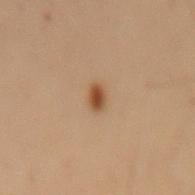Assessment:
The lesion was tiled from a total-body skin photograph and was not biopsied.
Image and clinical context:
A female patient roughly 50 years of age. The total-body-photography lesion software estimated a lesion area of about 3 mm², an eccentricity of roughly 0.8, and a shape-asymmetry score of about 0.2 (0 = symmetric). The software also gave a lesion color around L≈42 a*≈18 b*≈30 in CIELAB, roughly 11 lightness units darker than nearby skin, and a lesion-to-skin contrast of about 9 (normalized; higher = more distinct). And it measured radial color variation of about 0.5. Approximately 2.5 mm at its widest. Cropped from a total-body skin-imaging series; the visible field is about 15 mm. The lesion is located on the mid back. Captured under cross-polarized illumination.